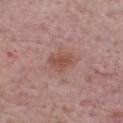  biopsy_status: not biopsied; imaged during a skin examination
  automated_metrics:
    color_variation_0_10: 2.5
    peripheral_color_asymmetry: 1.0
    nevus_likeness_0_100: 35
    lesion_detection_confidence_0_100: 100
  image:
    source: total-body photography crop
    field_of_view_mm: 15
  lesion_size:
    long_diameter_mm_approx: 3.0
  site: chest
  lighting: white-light
  patient:
    sex: male
    age_approx: 75